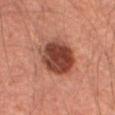{
  "biopsy_status": "not biopsied; imaged during a skin examination",
  "image": {
    "source": "total-body photography crop",
    "field_of_view_mm": 15
  },
  "automated_metrics": {
    "cielab_L": 37,
    "cielab_a": 23,
    "cielab_b": 26,
    "vs_skin_darker_L": 15.0,
    "vs_skin_contrast_norm": 11.5
  },
  "patient": {
    "sex": "male",
    "age_approx": 65
  },
  "site": "abdomen"
}